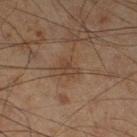Impression:
No biopsy was performed on this lesion — it was imaged during a full skin examination and was not determined to be concerning.
Background:
The patient is a male roughly 60 years of age. A 15 mm crop from a total-body photograph taken for skin-cancer surveillance. Located on the leg.